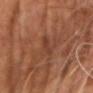Q: How was the tile lit?
A: cross-polarized illumination
Q: What is the imaging modality?
A: ~15 mm tile from a whole-body skin photo
Q: What did automated image analysis measure?
A: an automated nevus-likeness rating near 0 out of 100
Q: Patient demographics?
A: male, aged 58 to 62
Q: Where on the body is the lesion?
A: the upper back
Q: Lesion size?
A: ≈3 mm